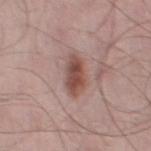notes = no biopsy performed (imaged during a skin exam)
illumination = white-light
subject = male, about 65 years old
anatomic site = the left thigh
image = ~15 mm crop, total-body skin-cancer survey